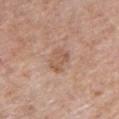The lesion was photographed on a routine skin check and not biopsied; there is no pathology result.
Cropped from a total-body skin-imaging series; the visible field is about 15 mm.
The lesion is on the front of the torso.
Imaged with white-light lighting.
A male patient aged 53 to 57.